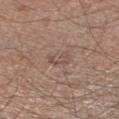Q: Was a biopsy performed?
A: imaged on a skin check; not biopsied
Q: What kind of image is this?
A: ~15 mm tile from a whole-body skin photo
Q: What is the anatomic site?
A: the right lower leg
Q: What did automated image analysis measure?
A: an area of roughly 3.5 mm²; a lesion color around L≈50 a*≈17 b*≈23 in CIELAB and about 6 CIELAB-L* units darker than the surrounding skin; a border-irregularity rating of about 5.5/10, a within-lesion color-variation index near 1.5/10, and a peripheral color-asymmetry measure near 0.5
Q: How large is the lesion?
A: about 2.5 mm
Q: What are the patient's age and sex?
A: male, aged 58 to 62
Q: Illumination type?
A: white-light illumination